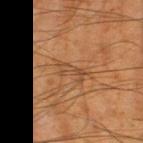The lesion was tiled from a total-body skin photograph and was not biopsied.
The lesion is on the left thigh.
Measured at roughly 3 mm in maximum diameter.
A close-up tile cropped from a whole-body skin photograph, about 15 mm across.
The patient is a male aged approximately 65.
This is a cross-polarized tile.